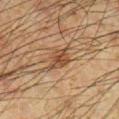| key | value |
|---|---|
| anatomic site | the right lower leg |
| patient | male, in their mid-30s |
| image | 15 mm crop, total-body photography |